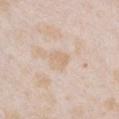Q: Was a biopsy performed?
A: imaged on a skin check; not biopsied
Q: What is the lesion's diameter?
A: ~2.5 mm (longest diameter)
Q: What kind of image is this?
A: total-body-photography crop, ~15 mm field of view
Q: What are the patient's age and sex?
A: female, about 25 years old
Q: How was the tile lit?
A: white-light
Q: Where on the body is the lesion?
A: the chest